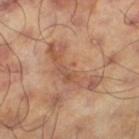Notes:
- workup: catalogued during a skin exam; not biopsied
- subject: male, aged 58–62
- lighting: cross-polarized
- image: total-body-photography crop, ~15 mm field of view
- site: the left thigh